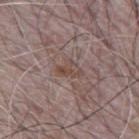Clinical impression:
No biopsy was performed on this lesion — it was imaged during a full skin examination and was not determined to be concerning.
Clinical summary:
This image is a 15 mm lesion crop taken from a total-body photograph. The subject is a male aged around 65. On the mid back.